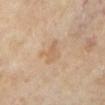The lesion was tiled from a total-body skin photograph and was not biopsied. This is a cross-polarized tile. A female subject, aged approximately 70. The lesion is located on the left lower leg. This image is a 15 mm lesion crop taken from a total-body photograph. Longest diameter approximately 3.5 mm. Automated image analysis of the tile measured a lesion area of about 6 mm² and a symmetry-axis asymmetry near 0.3. It also reported an average lesion color of about L≈64 a*≈17 b*≈34 (CIELAB) and a lesion-to-skin contrast of about 4.5 (normalized; higher = more distinct).